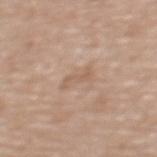The lesion is located on the upper back.
A female subject approximately 65 years of age.
A 15 mm crop from a total-body photograph taken for skin-cancer surveillance.
This is a white-light tile.
An algorithmic analysis of the crop reported a lesion color around L≈59 a*≈17 b*≈29 in CIELAB, a lesion–skin lightness drop of about 7, and a normalized lesion–skin contrast near 5.
About 3 mm across.